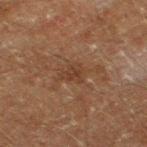Q: Was this lesion biopsied?
A: imaged on a skin check; not biopsied
Q: Lesion location?
A: the left thigh
Q: Who is the patient?
A: male, approximately 60 years of age
Q: What did automated image analysis measure?
A: a lesion–skin lightness drop of about 5; border irregularity of about 4 on a 0–10 scale, a within-lesion color-variation index near 1.5/10, and a peripheral color-asymmetry measure near 0.5
Q: How was this image acquired?
A: ~15 mm crop, total-body skin-cancer survey
Q: Lesion size?
A: ~2.5 mm (longest diameter)